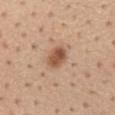Assessment: Imaged during a routine full-body skin examination; the lesion was not biopsied and no histopathology is available. Background: The lesion is on the back. A male subject, aged 53–57. The total-body-photography lesion software estimated a mean CIELAB color near L≈54 a*≈21 b*≈32 and about 13 CIELAB-L* units darker than the surrounding skin. The software also gave a border-irregularity index near 1.5/10 and radial color variation of about 1.5. And it measured a nevus-likeness score of about 95/100 and a detector confidence of about 100 out of 100 that the crop contains a lesion. A 15 mm close-up extracted from a 3D total-body photography capture. The recorded lesion diameter is about 3 mm. This is a white-light tile.